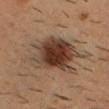| field | value |
|---|---|
| biopsy status | total-body-photography surveillance lesion; no biopsy |
| automated metrics | a footprint of about 24 mm², an outline eccentricity of about 0.7 (0 = round, 1 = elongated), and a shape-asymmetry score of about 0.25 (0 = symmetric); a lesion color around L≈34 a*≈15 b*≈24 in CIELAB, about 13 CIELAB-L* units darker than the surrounding skin, and a normalized border contrast of about 11.5; a border-irregularity index near 3.5/10 and a color-variation rating of about 6.5/10 |
| illumination | cross-polarized illumination |
| imaging modality | 15 mm crop, total-body photography |
| location | the head or neck |
| patient | male, aged 33–37 |
| diameter | ≈7 mm |